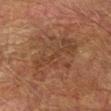Imaged during a routine full-body skin examination; the lesion was not biopsied and no histopathology is available. Imaged with cross-polarized lighting. A 15 mm close-up tile from a total-body photography series done for melanoma screening. From the left forearm. A male subject, aged approximately 75. Longest diameter approximately 8 mm.